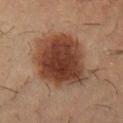Case summary:
• diameter · ≈8 mm
• site · the front of the torso
• subject · female, aged 28 to 32
• imaging modality · ~15 mm crop, total-body skin-cancer survey